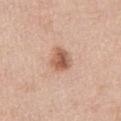Impression: Captured during whole-body skin photography for melanoma surveillance; the lesion was not biopsied. Background: The lesion-visualizer software estimated a footprint of about 6.5 mm², an outline eccentricity of about 0.6 (0 = round, 1 = elongated), and a symmetry-axis asymmetry near 0.25. The software also gave border irregularity of about 2.5 on a 0–10 scale, a color-variation rating of about 5.5/10, and a peripheral color-asymmetry measure near 2. The analysis additionally found a detector confidence of about 100 out of 100 that the crop contains a lesion. The lesion is on the abdomen. Longest diameter approximately 3 mm. The subject is a male aged around 60. The tile uses white-light illumination. Cropped from a whole-body photographic skin survey; the tile spans about 15 mm.